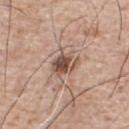biopsy_status: not biopsied; imaged during a skin examination
automated_metrics:
  area_mm2_approx: 6.5
  eccentricity: 0.6
  shape_asymmetry: 0.25
  nevus_likeness_0_100: 90
site: chest
lighting: white-light
image:
  source: total-body photography crop
  field_of_view_mm: 15
patient:
  sex: male
  age_approx: 75
lesion_size:
  long_diameter_mm_approx: 3.0